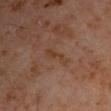Part of a total-body skin-imaging series; this lesion was reviewed on a skin check and was not flagged for biopsy. A male patient in their 60s. The lesion is on the left upper arm. The recorded lesion diameter is about 4 mm. The tile uses cross-polarized illumination. A close-up tile cropped from a whole-body skin photograph, about 15 mm across.